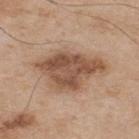- follow-up — catalogued during a skin exam; not biopsied
- acquisition — ~15 mm tile from a whole-body skin photo
- subject — male, aged 73–77
- site — the back
- tile lighting — white-light